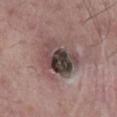Captured during whole-body skin photography for melanoma surveillance; the lesion was not biopsied. The total-body-photography lesion software estimated a lesion color around L≈42 a*≈14 b*≈17 in CIELAB, roughly 14 lightness units darker than nearby skin, and a lesion-to-skin contrast of about 11 (normalized; higher = more distinct). The analysis additionally found a border-irregularity rating of about 2.5/10, a color-variation rating of about 10/10, and peripheral color asymmetry of about 3.5. And it measured an automated nevus-likeness rating near 30 out of 100 and a detector confidence of about 100 out of 100 that the crop contains a lesion. The lesion is on the mid back. A male patient aged 63–67. The tile uses white-light illumination. A region of skin cropped from a whole-body photographic capture, roughly 15 mm wide.